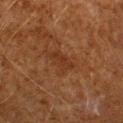notes=imaged on a skin check; not biopsied
subject=male, aged 58 to 62
lesion size=about 3 mm
image=total-body-photography crop, ~15 mm field of view
automated lesion analysis=a footprint of about 5 mm² and a symmetry-axis asymmetry near 0.25; a border-irregularity rating of about 3/10, a color-variation rating of about 1.5/10, and a peripheral color-asymmetry measure near 0.5; a classifier nevus-likeness of about 0/100 and a detector confidence of about 100 out of 100 that the crop contains a lesion
body site=the left forearm